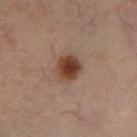Captured during whole-body skin photography for melanoma surveillance; the lesion was not biopsied. From the left lower leg. This image is a 15 mm lesion crop taken from a total-body photograph. Captured under cross-polarized illumination. The subject is a female roughly 55 years of age.